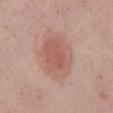Impression:
Captured during whole-body skin photography for melanoma surveillance; the lesion was not biopsied.
Image and clinical context:
A 15 mm crop from a total-body photograph taken for skin-cancer surveillance. A male patient approximately 60 years of age. The lesion is located on the abdomen. Captured under white-light illumination. The lesion-visualizer software estimated about 9 CIELAB-L* units darker than the surrounding skin and a lesion-to-skin contrast of about 6 (normalized; higher = more distinct). The software also gave border irregularity of about 2 on a 0–10 scale, a color-variation rating of about 4/10, and a peripheral color-asymmetry measure near 1. The lesion's longest dimension is about 6 mm.